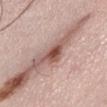Case summary:
• notes · imaged on a skin check; not biopsied
• imaging modality · total-body-photography crop, ~15 mm field of view
• subject · female, aged 48 to 52
• location · the abdomen
• size · ~4 mm (longest diameter)